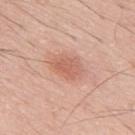This lesion was catalogued during total-body skin photography and was not selected for biopsy. The lesion's longest dimension is about 3.5 mm. On the mid back. A 15 mm close-up extracted from a 3D total-body photography capture. An algorithmic analysis of the crop reported a lesion area of about 7 mm², an eccentricity of roughly 0.7, and a symmetry-axis asymmetry near 0.2. It also reported an average lesion color of about L≈61 a*≈24 b*≈30 (CIELAB), about 9 CIELAB-L* units darker than the surrounding skin, and a normalized lesion–skin contrast near 6. The software also gave internal color variation of about 2.5 on a 0–10 scale and a peripheral color-asymmetry measure near 1. The patient is a male about 50 years old.